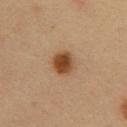follow-up: total-body-photography surveillance lesion; no biopsy
imaging modality: ~15 mm tile from a whole-body skin photo
diameter: ~3.5 mm (longest diameter)
subject: male, aged approximately 50
illumination: cross-polarized illumination
automated metrics: an area of roughly 7 mm², an eccentricity of roughly 0.6, and a shape-asymmetry score of about 0.15 (0 = symmetric); a mean CIELAB color near L≈46 a*≈21 b*≈35, roughly 14 lightness units darker than nearby skin, and a lesion-to-skin contrast of about 10.5 (normalized; higher = more distinct)
location: the abdomen